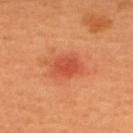Part of a total-body skin-imaging series; this lesion was reviewed on a skin check and was not flagged for biopsy.
The recorded lesion diameter is about 3.5 mm.
Captured under cross-polarized illumination.
Cropped from a total-body skin-imaging series; the visible field is about 15 mm.
A female subject in their 50s.